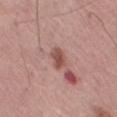follow-up=no biopsy performed (imaged during a skin exam) | patient=male, aged approximately 70 | tile lighting=white-light illumination | TBP lesion metrics=a footprint of about 4.5 mm², a shape eccentricity near 0.8, and two-axis asymmetry of about 0.2; roughly 11 lightness units darker than nearby skin | imaging modality=~15 mm crop, total-body skin-cancer survey | site=the left thigh.